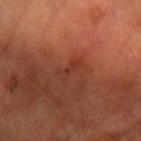{
  "site": "left forearm",
  "lighting": "cross-polarized",
  "lesion_size": {
    "long_diameter_mm_approx": 3.0
  },
  "patient": {
    "sex": "male",
    "age_approx": 65
  },
  "automated_metrics": {
    "cielab_L": 27,
    "cielab_a": 23,
    "cielab_b": 24,
    "vs_skin_darker_L": 4.0,
    "vs_skin_contrast_norm": 5.0,
    "lesion_detection_confidence_0_100": 65
  },
  "image": {
    "source": "total-body photography crop",
    "field_of_view_mm": 15
  }
}